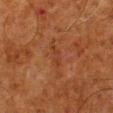Recorded during total-body skin imaging; not selected for excision or biopsy. A lesion tile, about 15 mm wide, cut from a 3D total-body photograph. On the left lower leg. The subject is a male about 80 years old.